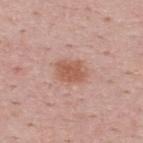Captured during whole-body skin photography for melanoma surveillance; the lesion was not biopsied. Located on the upper back. This is a white-light tile. Approximately 3.5 mm at its widest. A male patient, about 50 years old. Automated image analysis of the tile measured a mean CIELAB color near L≈57 a*≈23 b*≈29 and roughly 9 lightness units darker than nearby skin. A lesion tile, about 15 mm wide, cut from a 3D total-body photograph.